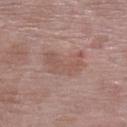<record>
<biopsy_status>not biopsied; imaged during a skin examination</biopsy_status>
</record>